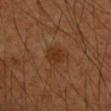{"biopsy_status": "not biopsied; imaged during a skin examination", "lighting": "cross-polarized", "site": "arm", "image": {"source": "total-body photography crop", "field_of_view_mm": 15}, "patient": {"sex": "male", "age_approx": 60}, "lesion_size": {"long_diameter_mm_approx": 2.5}}